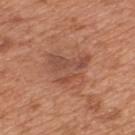Assessment: Recorded during total-body skin imaging; not selected for excision or biopsy. Acquisition and patient details: The subject is a male aged approximately 65. Captured under white-light illumination. Cropped from a total-body skin-imaging series; the visible field is about 15 mm. Located on the upper back.